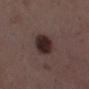About 3.5 mm across. Imaged with white-light lighting. A female subject aged 33–37. From the right lower leg. A lesion tile, about 15 mm wide, cut from a 3D total-body photograph.